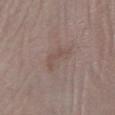Captured under white-light illumination.
Cropped from a whole-body photographic skin survey; the tile spans about 15 mm.
The lesion's longest dimension is about 3.5 mm.
On the leg.
The patient is a male aged 38 to 42.